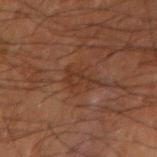Context:
The recorded lesion diameter is about 4 mm. On the left forearm. The subject is a male aged 68 to 72. Cropped from a whole-body photographic skin survey; the tile spans about 15 mm.